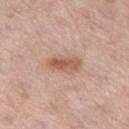Q: Is there a histopathology result?
A: total-body-photography surveillance lesion; no biopsy
Q: How was this image acquired?
A: 15 mm crop, total-body photography
Q: Where on the body is the lesion?
A: the left thigh
Q: Lesion size?
A: about 4 mm
Q: Automated lesion metrics?
A: a footprint of about 6.5 mm², an outline eccentricity of about 0.85 (0 = round, 1 = elongated), and two-axis asymmetry of about 0.2; an average lesion color of about L≈57 a*≈22 b*≈30 (CIELAB) and about 11 CIELAB-L* units darker than the surrounding skin; border irregularity of about 2.5 on a 0–10 scale and a peripheral color-asymmetry measure near 1.5; an automated nevus-likeness rating near 80 out of 100 and lesion-presence confidence of about 100/100
Q: What are the patient's age and sex?
A: male, aged approximately 60